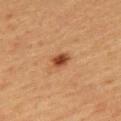This lesion was catalogued during total-body skin photography and was not selected for biopsy.
Captured under cross-polarized illumination.
A roughly 15 mm field-of-view crop from a total-body skin photograph.
A female subject, roughly 55 years of age.
From the upper back.